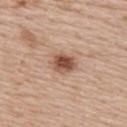biopsy status: catalogued during a skin exam; not biopsied
acquisition: 15 mm crop, total-body photography
illumination: white-light illumination
anatomic site: the back
diameter: ~3 mm (longest diameter)
subject: female, roughly 50 years of age